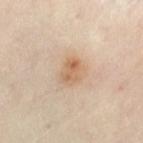A female subject, about 55 years old. The tile uses cross-polarized illumination. Measured at roughly 3 mm in maximum diameter. A 15 mm close-up extracted from a 3D total-body photography capture. The lesion is located on the left thigh.